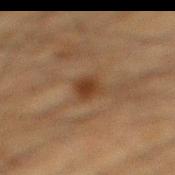This lesion was catalogued during total-body skin photography and was not selected for biopsy.
A male patient, approximately 35 years of age.
Longest diameter approximately 2.5 mm.
The lesion is on the leg.
Captured under cross-polarized illumination.
A 15 mm close-up extracted from a 3D total-body photography capture.